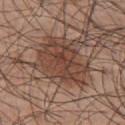The lesion was tiled from a total-body skin photograph and was not biopsied. From the chest. This image is a 15 mm lesion crop taken from a total-body photograph. A male patient, aged approximately 45. Imaged with white-light lighting. Approximately 7 mm at its widest. Automated tile analysis of the lesion measured a lesion–skin lightness drop of about 10 and a normalized border contrast of about 7.5. It also reported a border-irregularity rating of about 4/10 and radial color variation of about 1.5.